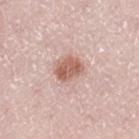Q: Is there a histopathology result?
A: no biopsy performed (imaged during a skin exam)
Q: Illumination type?
A: white-light
Q: What are the patient's age and sex?
A: male, aged around 50
Q: Lesion location?
A: the left thigh
Q: What is the lesion's diameter?
A: ~3 mm (longest diameter)
Q: Automated lesion metrics?
A: an average lesion color of about L≈60 a*≈22 b*≈26 (CIELAB), a lesion–skin lightness drop of about 13, and a normalized lesion–skin contrast near 8.5; border irregularity of about 1.5 on a 0–10 scale, a color-variation rating of about 3/10, and peripheral color asymmetry of about 1; an automated nevus-likeness rating near 90 out of 100 and a lesion-detection confidence of about 100/100
Q: What kind of image is this?
A: ~15 mm crop, total-body skin-cancer survey